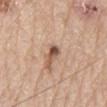notes: total-body-photography surveillance lesion; no biopsy | patient: male, aged 78 to 82 | diameter: about 2.5 mm | body site: the mid back | image source: ~15 mm crop, total-body skin-cancer survey | TBP lesion metrics: border irregularity of about 4 on a 0–10 scale, a within-lesion color-variation index near 1/10, and radial color variation of about 0; a classifier nevus-likeness of about 90/100 and a lesion-detection confidence of about 100/100.